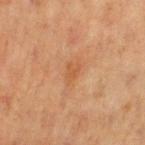| field | value |
|---|---|
| anatomic site | the left thigh |
| imaging modality | ~15 mm tile from a whole-body skin photo |
| illumination | cross-polarized |
| size | about 2.5 mm |
| automated lesion analysis | a mean CIELAB color near L≈48 a*≈22 b*≈34, about 6 CIELAB-L* units darker than the surrounding skin, and a normalized lesion–skin contrast near 5.5; a border-irregularity rating of about 2.5/10 and a peripheral color-asymmetry measure near 0.5 |
| patient | female, about 40 years old |